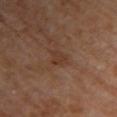notes = total-body-photography surveillance lesion; no biopsy
anatomic site = the upper back
automated lesion analysis = a lesion area of about 3.5 mm², an eccentricity of roughly 0.75, and a symmetry-axis asymmetry near 0.4; an average lesion color of about L≈37 a*≈19 b*≈28 (CIELAB), about 6 CIELAB-L* units darker than the surrounding skin, and a normalized lesion–skin contrast near 5.5; a nevus-likeness score of about 0/100
illumination = cross-polarized illumination
subject = female, roughly 65 years of age
diameter = ~2.5 mm (longest diameter)
acquisition = ~15 mm tile from a whole-body skin photo